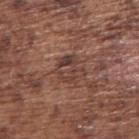| feature | finding |
|---|---|
| follow-up | total-body-photography surveillance lesion; no biopsy |
| patient | male, in their mid-70s |
| acquisition | ~15 mm tile from a whole-body skin photo |
| body site | the right upper arm |
| TBP lesion metrics | a lesion color around L≈40 a*≈19 b*≈24 in CIELAB and a lesion-to-skin contrast of about 7 (normalized; higher = more distinct); a border-irregularity rating of about 3.5/10, internal color variation of about 6.5 on a 0–10 scale, and a peripheral color-asymmetry measure near 2.5 |
| diameter | ~3.5 mm (longest diameter) |
| illumination | white-light illumination |